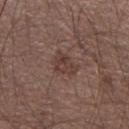Impression:
Captured during whole-body skin photography for melanoma surveillance; the lesion was not biopsied.
Clinical summary:
An algorithmic analysis of the crop reported a mean CIELAB color near L≈39 a*≈17 b*≈22. The software also gave a classifier nevus-likeness of about 5/100 and a lesion-detection confidence of about 100/100. A roughly 15 mm field-of-view crop from a total-body skin photograph. From the right upper arm. A male patient aged around 55. About 3.5 mm across. The tile uses white-light illumination.